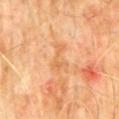Case summary:
* workup · total-body-photography surveillance lesion; no biopsy
* site · the front of the torso
* TBP lesion metrics · a footprint of about 5.5 mm² and an outline eccentricity of about 0.9 (0 = round, 1 = elongated); an average lesion color of about L≈64 a*≈24 b*≈42 (CIELAB) and a normalized lesion–skin contrast near 5
* size · about 4 mm
* patient · male, about 60 years old
* lighting · cross-polarized illumination
* imaging modality · 15 mm crop, total-body photography